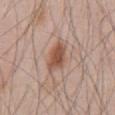<record>
<biopsy_status>not biopsied; imaged during a skin examination</biopsy_status>
<image>
  <source>total-body photography crop</source>
  <field_of_view_mm>15</field_of_view_mm>
</image>
<lighting>white-light</lighting>
<site>front of the torso</site>
<lesion_size>
  <long_diameter_mm_approx>4.0</long_diameter_mm_approx>
</lesion_size>
<patient>
  <sex>male</sex>
  <age_approx>45</age_approx>
</patient>
</record>